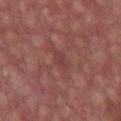Assessment:
Recorded during total-body skin imaging; not selected for excision or biopsy.
Acquisition and patient details:
The lesion is located on the chest. Automated image analysis of the tile measured an outline eccentricity of about 0.85 (0 = round, 1 = elongated) and a symmetry-axis asymmetry near 0.2. A region of skin cropped from a whole-body photographic capture, roughly 15 mm wide. The lesion's longest dimension is about 2.5 mm. A male patient roughly 60 years of age. Imaged with white-light lighting.